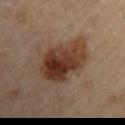Findings:
– workup · no biopsy performed (imaged during a skin exam)
– acquisition · ~15 mm crop, total-body skin-cancer survey
– anatomic site · the left upper arm
– tile lighting · cross-polarized illumination
– size · about 7 mm
– patient · male, approximately 85 years of age or older